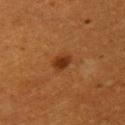The lesion was photographed on a routine skin check and not biopsied; there is no pathology result.
The lesion is located on the upper back.
A female subject about 55 years old.
A lesion tile, about 15 mm wide, cut from a 3D total-body photograph.